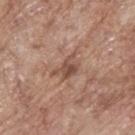Acquisition and patient details: The lesion's longest dimension is about 4 mm. On the upper back. The tile uses white-light illumination. A close-up tile cropped from a whole-body skin photograph, about 15 mm across. A male patient, approximately 70 years of age.